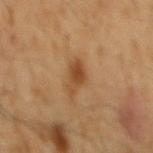biopsy status = no biopsy performed (imaged during a skin exam); patient = male, aged 58–62; tile lighting = cross-polarized illumination; anatomic site = the mid back; lesion diameter = ≈4 mm; acquisition = ~15 mm crop, total-body skin-cancer survey.